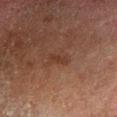Findings:
– workup · total-body-photography surveillance lesion; no biopsy
– lighting · cross-polarized
– body site · the left lower leg
– image-analysis metrics · a lesion area of about 2.5 mm², an outline eccentricity of about 0.95 (0 = round, 1 = elongated), and a shape-asymmetry score of about 0.3 (0 = symmetric); a within-lesion color-variation index near 0/10 and a peripheral color-asymmetry measure near 0; a detector confidence of about 100 out of 100 that the crop contains a lesion
– patient · male, in their 80s
– image · ~15 mm crop, total-body skin-cancer survey